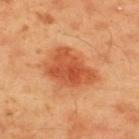<case>
<biopsy_status>not biopsied; imaged during a skin examination</biopsy_status>
<automated_metrics>
  <cielab_L>54</cielab_L>
  <cielab_a>32</cielab_a>
  <cielab_b>42</cielab_b>
  <border_irregularity_0_10>3.0</border_irregularity_0_10>
  <color_variation_0_10>4.0</color_variation_0_10>
  <peripheral_color_asymmetry>1.5</peripheral_color_asymmetry>
</automated_metrics>
<site>upper back</site>
<image>
  <source>total-body photography crop</source>
  <field_of_view_mm>15</field_of_view_mm>
</image>
<patient>
  <sex>male</sex>
  <age_approx>45</age_approx>
</patient>
<lighting>cross-polarized</lighting>
</case>